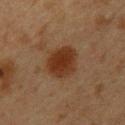The lesion was photographed on a routine skin check and not biopsied; there is no pathology result.
Located on the upper back.
A female patient, aged around 40.
Automated tile analysis of the lesion measured an area of roughly 12 mm², an eccentricity of roughly 0.5, and a shape-asymmetry score of about 0.15 (0 = symmetric). The software also gave a mean CIELAB color near L≈28 a*≈19 b*≈27 and roughly 9 lightness units darker than nearby skin. The software also gave an automated nevus-likeness rating near 100 out of 100.
A region of skin cropped from a whole-body photographic capture, roughly 15 mm wide.
The tile uses cross-polarized illumination.
Measured at roughly 4 mm in maximum diameter.